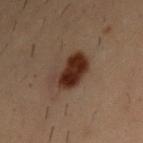notes = catalogued during a skin exam; not biopsied
acquisition = ~15 mm crop, total-body skin-cancer survey
location = the right upper arm
tile lighting = cross-polarized illumination
lesion diameter = ≈4 mm
patient = male, roughly 30 years of age